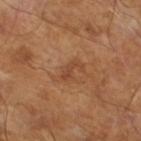{"automated_metrics": {"area_mm2_approx": 3.5, "eccentricity": 0.85, "nevus_likeness_0_100": 0}, "image": {"source": "total-body photography crop", "field_of_view_mm": 15}, "lighting": "cross-polarized", "patient": {"sex": "male", "age_approx": 65}, "lesion_size": {"long_diameter_mm_approx": 3.0}}